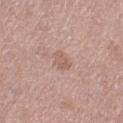  biopsy_status: not biopsied; imaged during a skin examination
  patient:
    sex: female
    age_approx: 50
  site: left thigh
  lesion_size:
    long_diameter_mm_approx: 2.5
  image:
    source: total-body photography crop
    field_of_view_mm: 15
  lighting: white-light